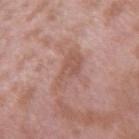Notes:
* workup — catalogued during a skin exam; not biopsied
* anatomic site — the arm
* acquisition — total-body-photography crop, ~15 mm field of view
* illumination — white-light
* automated lesion analysis — an area of roughly 8.5 mm² and an outline eccentricity of about 0.8 (0 = round, 1 = elongated); border irregularity of about 4.5 on a 0–10 scale, internal color variation of about 2.5 on a 0–10 scale, and radial color variation of about 1
* patient — male, aged approximately 40